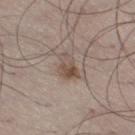Clinical impression:
Part of a total-body skin-imaging series; this lesion was reviewed on a skin check and was not flagged for biopsy.
Image and clinical context:
The patient is a male about 50 years old. On the leg. A 15 mm close-up tile from a total-body photography series done for melanoma screening.